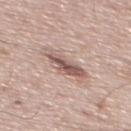Imaged during a routine full-body skin examination; the lesion was not biopsied and no histopathology is available.
Automated image analysis of the tile measured a lesion area of about 9 mm², an outline eccentricity of about 0.9 (0 = round, 1 = elongated), and a shape-asymmetry score of about 0.3 (0 = symmetric). It also reported radial color variation of about 2.5.
The recorded lesion diameter is about 5 mm.
A male subject in their mid- to late 60s.
The lesion is on the mid back.
Captured under white-light illumination.
A 15 mm close-up extracted from a 3D total-body photography capture.